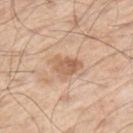The lesion was photographed on a routine skin check and not biopsied; there is no pathology result.
Located on the left upper arm.
A male subject about 70 years old.
The total-body-photography lesion software estimated a footprint of about 6.5 mm², an outline eccentricity of about 0.7 (0 = round, 1 = elongated), and two-axis asymmetry of about 0.35. And it measured a classifier nevus-likeness of about 5/100 and lesion-presence confidence of about 100/100.
This is a white-light tile.
This image is a 15 mm lesion crop taken from a total-body photograph.
Measured at roughly 3.5 mm in maximum diameter.